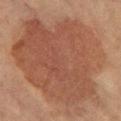This lesion was catalogued during total-body skin photography and was not selected for biopsy.
A female patient, aged 68 to 72.
An algorithmic analysis of the crop reported an average lesion color of about L≈49 a*≈21 b*≈30 (CIELAB), about 8 CIELAB-L* units darker than the surrounding skin, and a normalized border contrast of about 6.5. The software also gave a classifier nevus-likeness of about 55/100 and a lesion-detection confidence of about 100/100.
Cropped from a total-body skin-imaging series; the visible field is about 15 mm.
The tile uses cross-polarized illumination.
The lesion is located on the leg.
Approximately 16 mm at its widest.